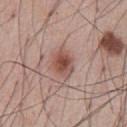{
  "biopsy_status": "not biopsied; imaged during a skin examination",
  "patient": {
    "sex": "male",
    "age_approx": 55
  },
  "lighting": "white-light",
  "lesion_size": {
    "long_diameter_mm_approx": 3.0
  },
  "image": {
    "source": "total-body photography crop",
    "field_of_view_mm": 15
  },
  "site": "abdomen",
  "automated_metrics": {
    "cielab_L": 50,
    "cielab_a": 23,
    "cielab_b": 25,
    "vs_skin_darker_L": 12.0,
    "vs_skin_contrast_norm": 8.5,
    "border_irregularity_0_10": 1.0,
    "color_variation_0_10": 4.5,
    "peripheral_color_asymmetry": 1.5,
    "nevus_likeness_0_100": 95,
    "lesion_detection_confidence_0_100": 100
  }
}